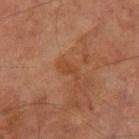Impression:
Recorded during total-body skin imaging; not selected for excision or biopsy.
Clinical summary:
A roughly 15 mm field-of-view crop from a total-body skin photograph. From the right thigh. This is a cross-polarized tile. The lesion-visualizer software estimated a lesion area of about 2.5 mm² and an outline eccentricity of about 0.9 (0 = round, 1 = elongated). The analysis additionally found an average lesion color of about L≈44 a*≈24 b*≈34 (CIELAB) and a lesion-to-skin contrast of about 5 (normalized; higher = more distinct). The analysis additionally found border irregularity of about 4.5 on a 0–10 scale, a within-lesion color-variation index near 0.5/10, and a peripheral color-asymmetry measure near 0. The software also gave a nevus-likeness score of about 0/100 and a detector confidence of about 100 out of 100 that the crop contains a lesion. A male patient, aged around 70.